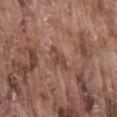notes: imaged on a skin check; not biopsied
subject: male, aged 73 to 77
TBP lesion metrics: an average lesion color of about L≈44 a*≈21 b*≈26 (CIELAB) and roughly 8 lightness units darker than nearby skin; lesion-presence confidence of about 100/100
tile lighting: white-light
image source: ~15 mm crop, total-body skin-cancer survey
anatomic site: the lower back